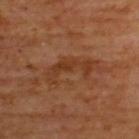Assessment:
The lesion was photographed on a routine skin check and not biopsied; there is no pathology result.
Image and clinical context:
Imaged with cross-polarized lighting. Approximately 6.5 mm at its widest. Cropped from a total-body skin-imaging series; the visible field is about 15 mm. Located on the upper back. A male patient aged 63 to 67. An algorithmic analysis of the crop reported an area of roughly 12 mm², an outline eccentricity of about 0.95 (0 = round, 1 = elongated), and a shape-asymmetry score of about 0.6 (0 = symmetric). The software also gave a mean CIELAB color near L≈37 a*≈22 b*≈32, about 6 CIELAB-L* units darker than the surrounding skin, and a normalized lesion–skin contrast near 6. The software also gave a nevus-likeness score of about 0/100.